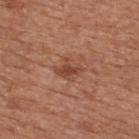Assessment:
No biopsy was performed on this lesion — it was imaged during a full skin examination and was not determined to be concerning.
Background:
The lesion is on the upper back. A lesion tile, about 15 mm wide, cut from a 3D total-body photograph. Captured under white-light illumination. A male subject, aged around 65.